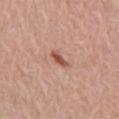Impression:
No biopsy was performed on this lesion — it was imaged during a full skin examination and was not determined to be concerning.
Acquisition and patient details:
A close-up tile cropped from a whole-body skin photograph, about 15 mm across. Measured at roughly 2.5 mm in maximum diameter. From the chest. An algorithmic analysis of the crop reported a footprint of about 3 mm², a shape eccentricity near 0.9, and two-axis asymmetry of about 0.35. And it measured an average lesion color of about L≈53 a*≈24 b*≈31 (CIELAB), roughly 12 lightness units darker than nearby skin, and a lesion-to-skin contrast of about 8.5 (normalized; higher = more distinct). The analysis additionally found a within-lesion color-variation index near 1/10 and peripheral color asymmetry of about 0. The software also gave an automated nevus-likeness rating near 85 out of 100 and a lesion-detection confidence of about 100/100. The subject is a male roughly 80 years of age.